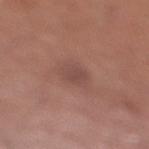biopsy status — total-body-photography surveillance lesion; no biopsy
image — 15 mm crop, total-body photography
patient — male, approximately 70 years of age
illumination — white-light
size — about 3 mm
anatomic site — the right lower leg